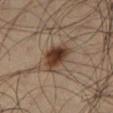{"biopsy_status": "not biopsied; imaged during a skin examination", "patient": {"sex": "male", "age_approx": 65}, "site": "leg", "image": {"source": "total-body photography crop", "field_of_view_mm": 15}, "lesion_size": {"long_diameter_mm_approx": 4.0}, "lighting": "cross-polarized"}